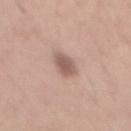| field | value |
|---|---|
| workup | catalogued during a skin exam; not biopsied |
| acquisition | total-body-photography crop, ~15 mm field of view |
| subject | male, aged around 30 |
| body site | the mid back |
| automated lesion analysis | a mean CIELAB color near L≈57 a*≈18 b*≈24 and a normalized border contrast of about 8; a border-irregularity rating of about 2/10, internal color variation of about 3 on a 0–10 scale, and peripheral color asymmetry of about 1; a nevus-likeness score of about 75/100 |
| tile lighting | white-light |
| diameter | about 3.5 mm |